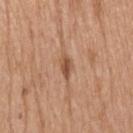workup — imaged on a skin check; not biopsied
subject — male, in their mid- to late 40s
acquisition — 15 mm crop, total-body photography
illumination — white-light illumination
anatomic site — the head or neck
lesion size — about 3 mm